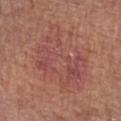Context:
An algorithmic analysis of the crop reported a footprint of about 29 mm², an outline eccentricity of about 0.75 (0 = round, 1 = elongated), and a shape-asymmetry score of about 0.4 (0 = symmetric). And it measured a within-lesion color-variation index near 5/10 and radial color variation of about 1.5. And it measured a classifier nevus-likeness of about 0/100 and lesion-presence confidence of about 100/100. The lesion's longest dimension is about 8 mm. From the right forearm. Cropped from a whole-body photographic skin survey; the tile spans about 15 mm. A female subject aged around 75. Captured under white-light illumination.